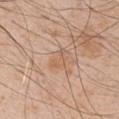biopsy status: no biopsy performed (imaged during a skin exam); image source: 15 mm crop, total-body photography; lighting: white-light; site: the left upper arm; lesion diameter: ~3 mm (longest diameter); subject: male, in their 50s.